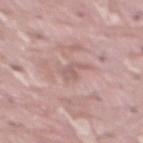Part of a total-body skin-imaging series; this lesion was reviewed on a skin check and was not flagged for biopsy. About 2.5 mm across. Cropped from a total-body skin-imaging series; the visible field is about 15 mm. A male patient in their 40s. The lesion is on the abdomen. Automated tile analysis of the lesion measured an average lesion color of about L≈59 a*≈19 b*≈21 (CIELAB), a lesion–skin lightness drop of about 8, and a normalized border contrast of about 5. The software also gave border irregularity of about 5.5 on a 0–10 scale, internal color variation of about 1 on a 0–10 scale, and a peripheral color-asymmetry measure near 0. The software also gave a nevus-likeness score of about 0/100.